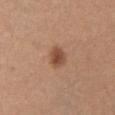Clinical impression:
Recorded during total-body skin imaging; not selected for excision or biopsy.
Image and clinical context:
Cropped from a total-body skin-imaging series; the visible field is about 15 mm. About 2.5 mm across. A female patient, aged approximately 45. The lesion is on the chest.